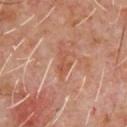<record>
<biopsy_status>not biopsied; imaged during a skin examination</biopsy_status>
<patient>
  <sex>male</sex>
  <age_approx>60</age_approx>
</patient>
<image>
  <source>total-body photography crop</source>
  <field_of_view_mm>15</field_of_view_mm>
</image>
<automated_metrics>
  <area_mm2_approx>3.0</area_mm2_approx>
  <eccentricity>0.9</eccentricity>
  <shape_asymmetry>0.4</shape_asymmetry>
  <border_irregularity_0_10>5.0</border_irregularity_0_10>
  <color_variation_0_10>0.0</color_variation_0_10>
  <peripheral_color_asymmetry>0.0</peripheral_color_asymmetry>
</automated_metrics>
<lesion_size>
  <long_diameter_mm_approx>3.0</long_diameter_mm_approx>
</lesion_size>
<site>chest</site>
</record>